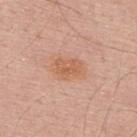notes: catalogued during a skin exam; not biopsied
image: 15 mm crop, total-body photography
diameter: ≈4 mm
patient: male, approximately 65 years of age
lighting: white-light
location: the upper back
TBP lesion metrics: a border-irregularity index near 2.5/10, a within-lesion color-variation index near 2.5/10, and peripheral color asymmetry of about 1